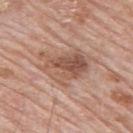Part of a total-body skin-imaging series; this lesion was reviewed on a skin check and was not flagged for biopsy. Automated image analysis of the tile measured a shape eccentricity near 0.7 and a symmetry-axis asymmetry near 0.3. It also reported a lesion color around L≈54 a*≈20 b*≈28 in CIELAB, about 10 CIELAB-L* units darker than the surrounding skin, and a lesion-to-skin contrast of about 7 (normalized; higher = more distinct). And it measured a border-irregularity index near 5/10, a within-lesion color-variation index near 7.5/10, and a peripheral color-asymmetry measure near 2.5. This is a white-light tile. Measured at roughly 5.5 mm in maximum diameter. This image is a 15 mm lesion crop taken from a total-body photograph. The lesion is located on the mid back. The patient is a male aged 78 to 82.